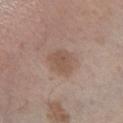The lesion-visualizer software estimated a footprint of about 7.5 mm², an eccentricity of roughly 0.5, and a symmetry-axis asymmetry near 0.25. The analysis additionally found internal color variation of about 2 on a 0–10 scale. The software also gave a nevus-likeness score of about 10/100 and a detector confidence of about 100 out of 100 that the crop contains a lesion. A male patient, aged approximately 60. The lesion's longest dimension is about 3.5 mm. Located on the leg. Captured under white-light illumination. A 15 mm crop from a total-body photograph taken for skin-cancer surveillance.